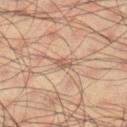workup=no biopsy performed (imaged during a skin exam); subject=male, about 35 years old; location=the right lower leg; size=~2.5 mm (longest diameter); tile lighting=cross-polarized; image=~15 mm crop, total-body skin-cancer survey.